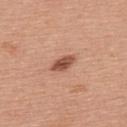Part of a total-body skin-imaging series; this lesion was reviewed on a skin check and was not flagged for biopsy.
Captured under white-light illumination.
A male patient aged 58 to 62.
Longest diameter approximately 3 mm.
From the upper back.
A region of skin cropped from a whole-body photographic capture, roughly 15 mm wide.